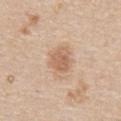Impression:
Part of a total-body skin-imaging series; this lesion was reviewed on a skin check and was not flagged for biopsy.
Context:
Imaged with white-light lighting. Cropped from a total-body skin-imaging series; the visible field is about 15 mm. The total-body-photography lesion software estimated border irregularity of about 2 on a 0–10 scale and radial color variation of about 1. On the abdomen. A male patient, about 65 years old. The lesion's longest dimension is about 3 mm.